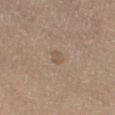Findings:
• follow-up: imaged on a skin check; not biopsied
• lighting: cross-polarized
• patient: female, in their mid- to late 40s
• site: the right thigh
• lesion size: ≈2.5 mm
• image: ~15 mm crop, total-body skin-cancer survey